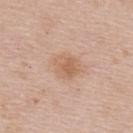Recorded during total-body skin imaging; not selected for excision or biopsy.
The patient is a female roughly 50 years of age.
From the upper back.
The lesion's longest dimension is about 3.5 mm.
Imaged with white-light lighting.
A close-up tile cropped from a whole-body skin photograph, about 15 mm across.